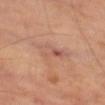{
  "biopsy_status": "not biopsied; imaged during a skin examination",
  "patient": {
    "sex": "male",
    "age_approx": 70
  },
  "site": "left thigh",
  "image": {
    "source": "total-body photography crop",
    "field_of_view_mm": 15
  }
}